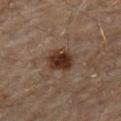{"biopsy_status": "not biopsied; imaged during a skin examination", "lesion_size": {"long_diameter_mm_approx": 3.5}, "patient": {"sex": "male", "age_approx": 45}, "image": {"source": "total-body photography crop", "field_of_view_mm": 15}, "site": "arm", "lighting": "cross-polarized"}